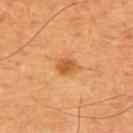notes = catalogued during a skin exam; not biopsied | tile lighting = cross-polarized | site = the back | automated metrics = a symmetry-axis asymmetry near 0.1; an average lesion color of about L≈47 a*≈23 b*≈39 (CIELAB), a lesion–skin lightness drop of about 9, and a normalized border contrast of about 7.5 | subject = male, roughly 65 years of age | imaging modality = 15 mm crop, total-body photography | size = ≈2.5 mm.